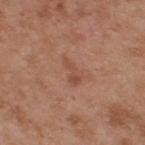<lesion>
<biopsy_status>not biopsied; imaged during a skin examination</biopsy_status>
<site>upper back</site>
<image>
  <source>total-body photography crop</source>
  <field_of_view_mm>15</field_of_view_mm>
</image>
<patient>
  <sex>male</sex>
  <age_approx>55</age_approx>
</patient>
<lesion_size>
  <long_diameter_mm_approx>3.0</long_diameter_mm_approx>
</lesion_size>
<automated_metrics>
  <area_mm2_approx>3.5</area_mm2_approx>
  <eccentricity>0.9</eccentricity>
  <shape_asymmetry>0.45</shape_asymmetry>
  <border_irregularity_0_10>5.0</border_irregularity_0_10>
  <color_variation_0_10>1.5</color_variation_0_10>
  <peripheral_color_asymmetry>0.5</peripheral_color_asymmetry>
</automated_metrics>
<lighting>white-light</lighting>
</lesion>